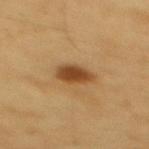Recorded during total-body skin imaging; not selected for excision or biopsy. A male patient aged approximately 60. The lesion is on the mid back. Automated image analysis of the tile measured an average lesion color of about L≈46 a*≈20 b*≈38 (CIELAB), a lesion–skin lightness drop of about 13, and a lesion-to-skin contrast of about 9.5 (normalized; higher = more distinct). Imaged with cross-polarized lighting. About 4 mm across. A roughly 15 mm field-of-view crop from a total-body skin photograph.